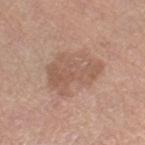Part of a total-body skin-imaging series; this lesion was reviewed on a skin check and was not flagged for biopsy. The subject is a female approximately 55 years of age. On the right thigh. A close-up tile cropped from a whole-body skin photograph, about 15 mm across.